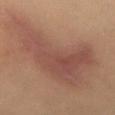Q: What lighting was used for the tile?
A: cross-polarized illumination
Q: What kind of image is this?
A: 15 mm crop, total-body photography
Q: Patient demographics?
A: female, aged around 40
Q: Where on the body is the lesion?
A: the abdomen
Q: What did automated image analysis measure?
A: a shape eccentricity near 0.85 and a shape-asymmetry score of about 0.45 (0 = symmetric); a mean CIELAB color near L≈39 a*≈17 b*≈22, about 7 CIELAB-L* units darker than the surrounding skin, and a normalized lesion–skin contrast near 7; a detector confidence of about 100 out of 100 that the crop contains a lesion